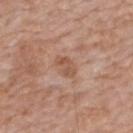Findings:
– notes — no biopsy performed (imaged during a skin exam)
– image — ~15 mm crop, total-body skin-cancer survey
– location — the mid back
– subject — male, aged 58–62